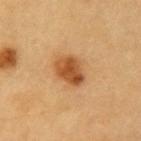biopsy_status: not biopsied; imaged during a skin examination
patient:
  sex: male
  age_approx: 60
lighting: cross-polarized
image:
  source: total-body photography crop
  field_of_view_mm: 15
lesion_size:
  long_diameter_mm_approx: 3.5
site: left upper arm